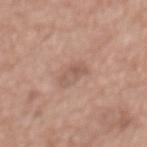Case summary:
* biopsy status — no biopsy performed (imaged during a skin exam)
* subject — female, aged approximately 75
* location — the mid back
* imaging modality — 15 mm crop, total-body photography